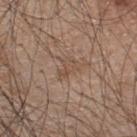Captured during whole-body skin photography for melanoma surveillance; the lesion was not biopsied. On the upper back. The lesion's longest dimension is about 3 mm. Imaged with white-light lighting. This image is a 15 mm lesion crop taken from a total-body photograph. A male patient, about 45 years old.